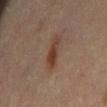follow-up = no biopsy performed (imaged during a skin exam).